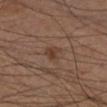Assessment:
Recorded during total-body skin imaging; not selected for excision or biopsy.
Clinical summary:
The tile uses cross-polarized illumination. The patient is a male roughly 55 years of age. Measured at roughly 2.5 mm in maximum diameter. The lesion is located on the left lower leg. This image is a 15 mm lesion crop taken from a total-body photograph. Automated tile analysis of the lesion measured an area of roughly 3.5 mm², a shape eccentricity near 0.65, and a symmetry-axis asymmetry near 0.4. The analysis additionally found a lesion color around L≈38 a*≈17 b*≈26 in CIELAB and a normalized lesion–skin contrast near 6.5. And it measured a border-irregularity rating of about 3.5/10, internal color variation of about 2 on a 0–10 scale, and radial color variation of about 0.5.